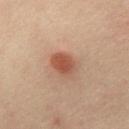Captured during whole-body skin photography for melanoma surveillance; the lesion was not biopsied. A male patient about 65 years old. On the front of the torso. A roughly 15 mm field-of-view crop from a total-body skin photograph. Automated image analysis of the tile measured border irregularity of about 1.5 on a 0–10 scale, a within-lesion color-variation index near 4.5/10, and a peripheral color-asymmetry measure near 1.5.